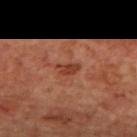The lesion was tiled from a total-body skin photograph and was not biopsied. Approximately 2.5 mm at its widest. Imaged with cross-polarized lighting. Located on the mid back. A male patient, aged 68 to 72. Cropped from a whole-body photographic skin survey; the tile spans about 15 mm.